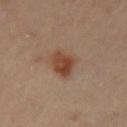{"site": "left upper arm", "lesion_size": {"long_diameter_mm_approx": 4.0}, "lighting": "cross-polarized", "patient": {"sex": "female", "age_approx": 45}, "image": {"source": "total-body photography crop", "field_of_view_mm": 15}, "automated_metrics": {"border_irregularity_0_10": 2.0, "color_variation_0_10": 4.5, "peripheral_color_asymmetry": 1.5}}